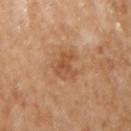workup = no biopsy performed (imaged during a skin exam); acquisition = 15 mm crop, total-body photography; location = the arm; subject = female, in their 60s.